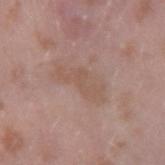<record>
  <biopsy_status>not biopsied; imaged during a skin examination</biopsy_status>
  <site>right upper arm</site>
  <image>
    <source>total-body photography crop</source>
    <field_of_view_mm>15</field_of_view_mm>
  </image>
  <patient>
    <sex>male</sex>
    <age_approx>40</age_approx>
  </patient>
  <lighting>white-light</lighting>
</record>